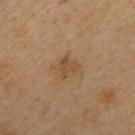Case summary:
– patient · male, aged 38–42
– anatomic site · the upper back
– tile lighting · cross-polarized
– image source · ~15 mm tile from a whole-body skin photo
– diameter · ≈2.5 mm